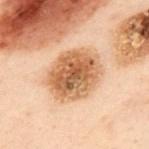The lesion was tiled from a total-body skin photograph and was not biopsied.
Longest diameter approximately 6 mm.
Located on the upper back.
A 15 mm close-up tile from a total-body photography series done for melanoma screening.
Imaged with cross-polarized lighting.
A male patient roughly 50 years of age.